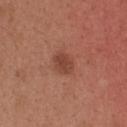follow-up: catalogued during a skin exam; not biopsied | illumination: white-light illumination | subject: female, aged 28–32 | image: ~15 mm tile from a whole-body skin photo | body site: the upper back | size: about 3 mm.